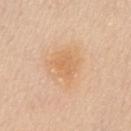workup: total-body-photography surveillance lesion; no biopsy
body site: the chest
patient: female, in their mid-50s
acquisition: total-body-photography crop, ~15 mm field of view